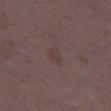{"biopsy_status": "not biopsied; imaged during a skin examination", "lighting": "white-light", "patient": {"sex": "female", "age_approx": 35}, "automated_metrics": {"area_mm2_approx": 2.5, "eccentricity": 0.85, "cielab_L": 38, "cielab_a": 14, "cielab_b": 19, "vs_skin_darker_L": 4.0, "nevus_likeness_0_100": 0}, "image": {"source": "total-body photography crop", "field_of_view_mm": 15}, "site": "right thigh", "lesion_size": {"long_diameter_mm_approx": 2.5}}